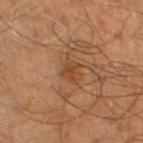Q: What are the patient's age and sex?
A: male, aged around 70
Q: What is the anatomic site?
A: the right thigh
Q: What is the imaging modality?
A: ~15 mm tile from a whole-body skin photo